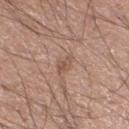Clinical impression:
No biopsy was performed on this lesion — it was imaged during a full skin examination and was not determined to be concerning.
Context:
Cropped from a whole-body photographic skin survey; the tile spans about 15 mm. The subject is a male aged around 20. Imaged with white-light lighting. Approximately 2.5 mm at its widest. From the left lower leg.